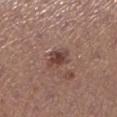Clinical impression:
This lesion was catalogued during total-body skin photography and was not selected for biopsy.
Image and clinical context:
The tile uses white-light illumination. Measured at roughly 3.5 mm in maximum diameter. On the leg. A female patient, roughly 25 years of age. A 15 mm crop from a total-body photograph taken for skin-cancer surveillance.